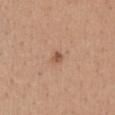biopsy status=catalogued during a skin exam; not biopsied
tile lighting=white-light
imaging modality=~15 mm tile from a whole-body skin photo
body site=the chest
TBP lesion metrics=an area of roughly 2 mm², a shape eccentricity near 0.5, and two-axis asymmetry of about 0.25; peripheral color asymmetry of about 0.5
subject=female, about 45 years old
diameter=~1.5 mm (longest diameter)